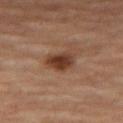<lesion>
  <biopsy_status>not biopsied; imaged during a skin examination</biopsy_status>
  <image>
    <source>total-body photography crop</source>
    <field_of_view_mm>15</field_of_view_mm>
  </image>
  <site>leg</site>
  <lighting>cross-polarized</lighting>
  <lesion_size>
    <long_diameter_mm_approx>4.0</long_diameter_mm_approx>
  </lesion_size>
  <patient>
    <sex>female</sex>
    <age_approx>65</age_approx>
  </patient>
  <automated_metrics>
    <area_mm2_approx>8.0</area_mm2_approx>
    <eccentricity>0.7</eccentricity>
    <shape_asymmetry>0.25</shape_asymmetry>
    <cielab_L>37</cielab_L>
    <cielab_a>20</cielab_a>
    <cielab_b>28</cielab_b>
    <vs_skin_contrast_norm>10.0</vs_skin_contrast_norm>
    <nevus_likeness_0_100>95</nevus_likeness_0_100>
    <lesion_detection_confidence_0_100>100</lesion_detection_confidence_0_100>
  </automated_metrics>
</lesion>